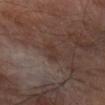The total-body-photography lesion software estimated a lesion area of about 3.5 mm², a shape eccentricity near 0.85, and two-axis asymmetry of about 0.4. The analysis additionally found a mean CIELAB color near L≈32 a*≈16 b*≈20, about 5 CIELAB-L* units darker than the surrounding skin, and a lesion-to-skin contrast of about 5 (normalized; higher = more distinct). It also reported a nevus-likeness score of about 0/100 and a detector confidence of about 100 out of 100 that the crop contains a lesion.
On the arm.
A region of skin cropped from a whole-body photographic capture, roughly 15 mm wide.
A male patient in their 70s.